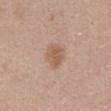Impression:
This lesion was catalogued during total-body skin photography and was not selected for biopsy.
Acquisition and patient details:
A female subject aged 38–42. A close-up tile cropped from a whole-body skin photograph, about 15 mm across. This is a white-light tile. The lesion is located on the abdomen. Measured at roughly 3 mm in maximum diameter.